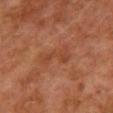Part of a total-body skin-imaging series; this lesion was reviewed on a skin check and was not flagged for biopsy. The patient is a female approximately 60 years of age. This is a cross-polarized tile. The lesion-visualizer software estimated a footprint of about 7 mm², an outline eccentricity of about 0.9 (0 = round, 1 = elongated), and two-axis asymmetry of about 0.4. The software also gave border irregularity of about 5.5 on a 0–10 scale and internal color variation of about 2 on a 0–10 scale. On the chest. Measured at roughly 4 mm in maximum diameter. This image is a 15 mm lesion crop taken from a total-body photograph.